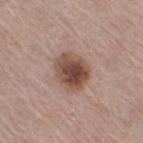Impression:
Recorded during total-body skin imaging; not selected for excision or biopsy.
Image and clinical context:
Captured under white-light illumination. A 15 mm close-up tile from a total-body photography series done for melanoma screening. Measured at roughly 4.5 mm in maximum diameter. A female subject, aged approximately 40. The lesion is on the right thigh. The lesion-visualizer software estimated a lesion area of about 13 mm². The software also gave a border-irregularity index near 1.5/10 and peripheral color asymmetry of about 2. The analysis additionally found an automated nevus-likeness rating near 95 out of 100 and lesion-presence confidence of about 100/100.